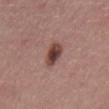Findings:
- workup: no biopsy performed (imaged during a skin exam)
- tile lighting: white-light
- TBP lesion metrics: a mean CIELAB color near L≈42 a*≈22 b*≈21, roughly 14 lightness units darker than nearby skin, and a normalized border contrast of about 11; a border-irregularity rating of about 1.5/10, a within-lesion color-variation index near 5/10, and a peripheral color-asymmetry measure near 1.5; an automated nevus-likeness rating near 95 out of 100 and lesion-presence confidence of about 100/100
- diameter: ~3.5 mm (longest diameter)
- image source: ~15 mm tile from a whole-body skin photo
- subject: male, aged around 40
- anatomic site: the abdomen